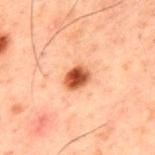Clinical impression: Captured during whole-body skin photography for melanoma surveillance; the lesion was not biopsied. Image and clinical context: A roughly 15 mm field-of-view crop from a total-body skin photograph. The lesion is located on the upper back. A male patient roughly 60 years of age.